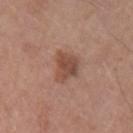No biopsy was performed on this lesion — it was imaged during a full skin examination and was not determined to be concerning. A male subject aged 53 to 57. Automated image analysis of the tile measured a footprint of about 8 mm² and an eccentricity of roughly 0.45. The software also gave a border-irregularity index near 3.5/10, a color-variation rating of about 3.5/10, and a peripheral color-asymmetry measure near 1. The analysis additionally found an automated nevus-likeness rating near 40 out of 100 and a detector confidence of about 100 out of 100 that the crop contains a lesion. The lesion's longest dimension is about 3.5 mm. From the left upper arm. Cropped from a total-body skin-imaging series; the visible field is about 15 mm.